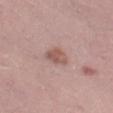size = ~3 mm (longest diameter); tile lighting = white-light illumination; subject = male, aged around 30; acquisition = ~15 mm tile from a whole-body skin photo; automated lesion analysis = a border-irregularity rating of about 2/10, internal color variation of about 2 on a 0–10 scale, and peripheral color asymmetry of about 1.